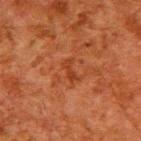Impression: Captured during whole-body skin photography for melanoma surveillance; the lesion was not biopsied. Background: Cropped from a total-body skin-imaging series; the visible field is about 15 mm. The patient is a male aged 58 to 62. Located on the upper back. This is a cross-polarized tile.